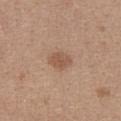  biopsy_status: not biopsied; imaged during a skin examination
  automated_metrics:
    border_irregularity_0_10: 1.5
    color_variation_0_10: 2.0
    peripheral_color_asymmetry: 0.5
    lesion_detection_confidence_0_100: 100
  patient:
    sex: female
    age_approx: 35
  lighting: white-light
  lesion_size:
    long_diameter_mm_approx: 3.0
  image:
    source: total-body photography crop
    field_of_view_mm: 15
  site: abdomen